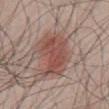patient: male, roughly 50 years of age
body site: the abdomen
tile lighting: white-light illumination
acquisition: total-body-photography crop, ~15 mm field of view